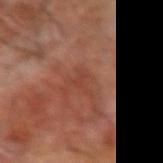Q: Was a biopsy performed?
A: total-body-photography surveillance lesion; no biopsy
Q: How was the tile lit?
A: cross-polarized illumination
Q: Patient demographics?
A: male, roughly 65 years of age
Q: What did automated image analysis measure?
A: an average lesion color of about L≈41 a*≈25 b*≈29 (CIELAB), a lesion–skin lightness drop of about 5, and a lesion-to-skin contrast of about 4.5 (normalized; higher = more distinct); a border-irregularity rating of about 7.5/10, a within-lesion color-variation index near 0.5/10, and a peripheral color-asymmetry measure near 0.5
Q: What is the imaging modality?
A: ~15 mm tile from a whole-body skin photo
Q: Lesion location?
A: the right forearm